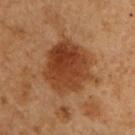Clinical impression:
Part of a total-body skin-imaging series; this lesion was reviewed on a skin check and was not flagged for biopsy.
Background:
From the chest. Imaged with cross-polarized lighting. A male subject, roughly 50 years of age. A 15 mm close-up extracted from a 3D total-body photography capture. Approximately 6.5 mm at its widest.